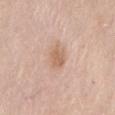Acquisition and patient details: An algorithmic analysis of the crop reported an area of roughly 5.5 mm², an outline eccentricity of about 0.6 (0 = round, 1 = elongated), and a shape-asymmetry score of about 0.15 (0 = symmetric). The software also gave roughly 8 lightness units darker than nearby skin. The analysis additionally found a nevus-likeness score of about 50/100 and a lesion-detection confidence of about 100/100. Located on the back. Cropped from a whole-body photographic skin survey; the tile spans about 15 mm. The patient is a female approximately 65 years of age. Approximately 3 mm at its widest.